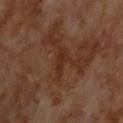Q: Was this lesion biopsied?
A: catalogued during a skin exam; not biopsied
Q: How was this image acquired?
A: ~15 mm tile from a whole-body skin photo
Q: Lesion location?
A: the back
Q: Patient demographics?
A: male, about 70 years old